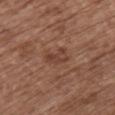notes: no biopsy performed (imaged during a skin exam) | site: the chest | patient: female, approximately 75 years of age | size: about 3 mm | automated lesion analysis: a footprint of about 4.5 mm² and a shape-asymmetry score of about 0.35 (0 = symmetric); an average lesion color of about L≈41 a*≈21 b*≈27 (CIELAB), about 7 CIELAB-L* units darker than the surrounding skin, and a normalized lesion–skin contrast near 6 | imaging modality: ~15 mm crop, total-body skin-cancer survey.